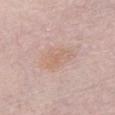Impression: The lesion was tiled from a total-body skin photograph and was not biopsied. Acquisition and patient details: Located on the chest. A 15 mm crop from a total-body photograph taken for skin-cancer surveillance. The recorded lesion diameter is about 4.5 mm. The subject is a female approximately 60 years of age. Imaged with white-light lighting.